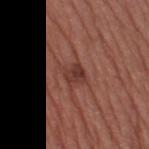Recorded during total-body skin imaging; not selected for excision or biopsy. The subject is a female aged around 40. The tile uses white-light illumination. Located on the right thigh. Approximately 2.5 mm at its widest. A 15 mm crop from a total-body photograph taken for skin-cancer surveillance. An algorithmic analysis of the crop reported a lesion area of about 5 mm², an eccentricity of roughly 0.3, and a symmetry-axis asymmetry near 0.3. The software also gave a mean CIELAB color near L≈37 a*≈24 b*≈25, a lesion–skin lightness drop of about 9, and a normalized lesion–skin contrast near 7.5. It also reported a color-variation rating of about 4/10 and a peripheral color-asymmetry measure near 1.5. It also reported an automated nevus-likeness rating near 20 out of 100 and a lesion-detection confidence of about 100/100.